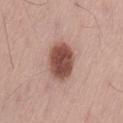| key | value |
|---|---|
| follow-up | catalogued during a skin exam; not biopsied |
| image | 15 mm crop, total-body photography |
| patient | male, roughly 55 years of age |
| location | the lower back |
| lighting | white-light illumination |
| lesion diameter | ≈4.5 mm |